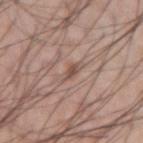The lesion was tiled from a total-body skin photograph and was not biopsied.
This is a white-light tile.
A male patient, aged around 50.
The lesion's longest dimension is about 3 mm.
The lesion is on the right upper arm.
The lesion-visualizer software estimated a lesion-to-skin contrast of about 6.5 (normalized; higher = more distinct). The analysis additionally found a within-lesion color-variation index near 0/10. It also reported a nevus-likeness score of about 0/100 and lesion-presence confidence of about 70/100.
A 15 mm close-up tile from a total-body photography series done for melanoma screening.